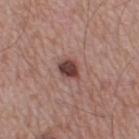Imaged during a routine full-body skin examination; the lesion was not biopsied and no histopathology is available. A 15 mm crop from a total-body photograph taken for skin-cancer surveillance. From the right lower leg. A male patient, in their mid- to late 50s. An algorithmic analysis of the crop reported a shape eccentricity near 0.65 and a shape-asymmetry score of about 0.2 (0 = symmetric). The analysis additionally found roughly 15 lightness units darker than nearby skin. The analysis additionally found a border-irregularity index near 2/10, a color-variation rating of about 4.5/10, and a peripheral color-asymmetry measure near 1.5.